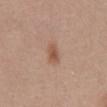follow-up=total-body-photography surveillance lesion; no biopsy
subject=female, aged 38 to 42
lighting=white-light illumination
imaging modality=~15 mm tile from a whole-body skin photo
size=about 3 mm
automated metrics=a border-irregularity index near 2/10, a color-variation rating of about 2.5/10, and a peripheral color-asymmetry measure near 1
site=the abdomen